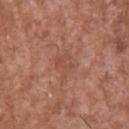Q: Is there a histopathology result?
A: no biopsy performed (imaged during a skin exam)
Q: Who is the patient?
A: male, roughly 45 years of age
Q: How large is the lesion?
A: about 3.5 mm
Q: What is the imaging modality?
A: 15 mm crop, total-body photography
Q: What is the anatomic site?
A: the upper back
Q: Illumination type?
A: white-light